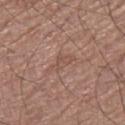The lesion was tiled from a total-body skin photograph and was not biopsied. The subject is a male in their mid- to late 70s. The lesion is located on the right thigh. Captured under white-light illumination. The recorded lesion diameter is about 3 mm. A 15 mm crop from a total-body photograph taken for skin-cancer surveillance. An algorithmic analysis of the crop reported a lesion area of about 4 mm², an outline eccentricity of about 0.85 (0 = round, 1 = elongated), and a symmetry-axis asymmetry near 0.35. The analysis additionally found roughly 6 lightness units darker than nearby skin and a normalized border contrast of about 4.5.